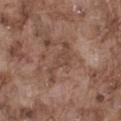biopsy status — total-body-photography surveillance lesion; no biopsy
imaging modality — ~15 mm tile from a whole-body skin photo
site — the abdomen
image-analysis metrics — a classifier nevus-likeness of about 0/100 and lesion-presence confidence of about 60/100
subject — male, roughly 75 years of age